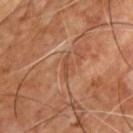This is a cross-polarized tile. Cropped from a total-body skin-imaging series; the visible field is about 15 mm. The lesion's longest dimension is about 2.5 mm. The lesion is located on the front of the torso. A male subject, aged around 55.